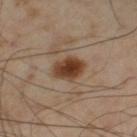{"biopsy_status": "not biopsied; imaged during a skin examination", "patient": {"sex": "male", "age_approx": 55}, "lesion_size": {"long_diameter_mm_approx": 4.0}, "lighting": "cross-polarized", "image": {"source": "total-body photography crop", "field_of_view_mm": 15}, "site": "leg"}